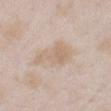Q: What are the patient's age and sex?
A: female, aged 23–27
Q: How was this image acquired?
A: 15 mm crop, total-body photography
Q: Lesion location?
A: the left forearm
Q: Illumination type?
A: white-light
Q: What is the lesion's diameter?
A: ~5 mm (longest diameter)